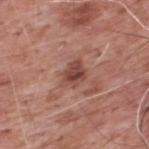biopsy_status: not biopsied; imaged during a skin examination
lesion_size:
  long_diameter_mm_approx: 3.5
site: upper back
patient:
  sex: male
  age_approx: 60
lighting: white-light
image:
  source: total-body photography crop
  field_of_view_mm: 15